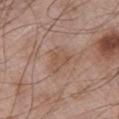Findings:
* notes — catalogued during a skin exam; not biopsied
* body site — the chest
* tile lighting — white-light illumination
* patient — male, in their mid- to late 60s
* image-analysis metrics — a lesion area of about 6.5 mm², an eccentricity of roughly 0.6, and two-axis asymmetry of about 0.4; an average lesion color of about L≈53 a*≈18 b*≈28 (CIELAB), about 6 CIELAB-L* units darker than the surrounding skin, and a normalized lesion–skin contrast near 5.5; a classifier nevus-likeness of about 0/100 and a detector confidence of about 100 out of 100 that the crop contains a lesion
* acquisition — total-body-photography crop, ~15 mm field of view